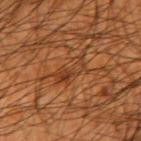Recorded during total-body skin imaging; not selected for excision or biopsy. The lesion is on the right upper arm. A region of skin cropped from a whole-body photographic capture, roughly 15 mm wide. The tile uses cross-polarized illumination. A male subject, aged approximately 45. The lesion's longest dimension is about 4.5 mm.